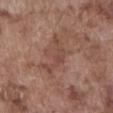Notes:
– notes — catalogued during a skin exam; not biopsied
– size — ~5.5 mm (longest diameter)
– lighting — white-light
– patient — male, aged 73 to 77
– TBP lesion metrics — an area of roughly 7 mm² and a symmetry-axis asymmetry near 0.65; border irregularity of about 9 on a 0–10 scale and a peripheral color-asymmetry measure near 0.5
– location — the abdomen
– imaging modality — ~15 mm tile from a whole-body skin photo